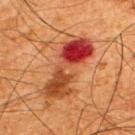Clinical impression:
Recorded during total-body skin imaging; not selected for excision or biopsy.
Image and clinical context:
The subject is a male in their mid- to late 60s. The lesion is located on the upper back. This is a cross-polarized tile. A region of skin cropped from a whole-body photographic capture, roughly 15 mm wide. The lesion's longest dimension is about 8.5 mm.